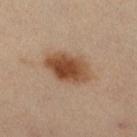follow-up: no biopsy performed (imaged during a skin exam).